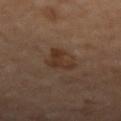Part of a total-body skin-imaging series; this lesion was reviewed on a skin check and was not flagged for biopsy.
A female subject, aged 58–62.
Measured at roughly 3.5 mm in maximum diameter.
Imaged with cross-polarized lighting.
The lesion is located on the left forearm.
A lesion tile, about 15 mm wide, cut from a 3D total-body photograph.